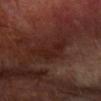Case summary:
* illumination · cross-polarized illumination
* subject · male, roughly 70 years of age
* size · about 5 mm
* location · the right forearm
* image · total-body-photography crop, ~15 mm field of view
* TBP lesion metrics · a lesion color around L≈22 a*≈20 b*≈22 in CIELAB; an automated nevus-likeness rating near 0 out of 100 and a lesion-detection confidence of about 70/100